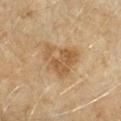Q: Was a biopsy performed?
A: no biopsy performed (imaged during a skin exam)
Q: How was this image acquired?
A: ~15 mm tile from a whole-body skin photo
Q: What is the lesion's diameter?
A: ≈5 mm
Q: Lesion location?
A: the left forearm
Q: What lighting was used for the tile?
A: cross-polarized
Q: What did automated image analysis measure?
A: a lesion-to-skin contrast of about 7 (normalized; higher = more distinct); a border-irregularity rating of about 7/10, a color-variation rating of about 3/10, and peripheral color asymmetry of about 1; a nevus-likeness score of about 10/100
Q: Who is the patient?
A: female, aged 58–62